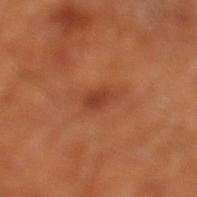Q: Is there a histopathology result?
A: imaged on a skin check; not biopsied
Q: How large is the lesion?
A: about 2.5 mm
Q: What is the imaging modality?
A: ~15 mm tile from a whole-body skin photo
Q: What did automated image analysis measure?
A: an area of roughly 3.5 mm²; an average lesion color of about L≈40 a*≈28 b*≈34 (CIELAB) and about 8 CIELAB-L* units darker than the surrounding skin
Q: What are the patient's age and sex?
A: aged 63–67
Q: What lighting was used for the tile?
A: cross-polarized illumination
Q: What is the anatomic site?
A: the left lower leg